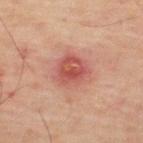follow-up = imaged on a skin check; not biopsied | illumination = cross-polarized | image source = ~15 mm crop, total-body skin-cancer survey | patient = male, aged around 60 | body site = the back | lesion size = ~4 mm (longest diameter).